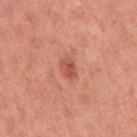Assessment: Imaged during a routine full-body skin examination; the lesion was not biopsied and no histopathology is available. Acquisition and patient details: A close-up tile cropped from a whole-body skin photograph, about 15 mm across. From the arm. A female patient, about 50 years old.